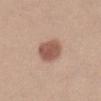Recorded during total-body skin imaging; not selected for excision or biopsy.
On the abdomen.
About 3.5 mm across.
A lesion tile, about 15 mm wide, cut from a 3D total-body photograph.
The subject is a female about 25 years old.